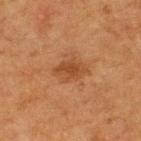Q: Was a biopsy performed?
A: total-body-photography surveillance lesion; no biopsy
Q: What did automated image analysis measure?
A: a footprint of about 7 mm² and an eccentricity of roughly 0.75; roughly 7 lightness units darker than nearby skin and a normalized lesion–skin contrast near 6.5; an automated nevus-likeness rating near 55 out of 100 and a lesion-detection confidence of about 100/100
Q: How was the tile lit?
A: cross-polarized illumination
Q: Patient demographics?
A: male, aged approximately 75
Q: Lesion location?
A: the back
Q: Lesion size?
A: ≈4 mm
Q: What kind of image is this?
A: ~15 mm tile from a whole-body skin photo